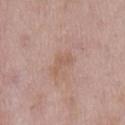Impression: Imaged during a routine full-body skin examination; the lesion was not biopsied and no histopathology is available. Context: The tile uses white-light illumination. The recorded lesion diameter is about 3 mm. The patient is a male in their mid-50s. Located on the mid back. The lesion-visualizer software estimated a border-irregularity rating of about 5.5/10, a within-lesion color-variation index near 0.5/10, and radial color variation of about 0. A 15 mm crop from a total-body photograph taken for skin-cancer surveillance.